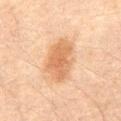No biopsy was performed on this lesion — it was imaged during a full skin examination and was not determined to be concerning. A close-up tile cropped from a whole-body skin photograph, about 15 mm across. A male patient aged 58–62. Captured under cross-polarized illumination. The recorded lesion diameter is about 5.5 mm. The lesion is on the abdomen. An algorithmic analysis of the crop reported a shape-asymmetry score of about 0.2 (0 = symmetric). The software also gave roughly 9 lightness units darker than nearby skin and a normalized lesion–skin contrast near 7. And it measured border irregularity of about 2 on a 0–10 scale and a color-variation rating of about 3/10.